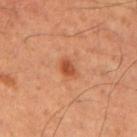Findings:
– follow-up — catalogued during a skin exam; not biopsied
– acquisition — total-body-photography crop, ~15 mm field of view
– body site — the arm
– lesion diameter — ≈2.5 mm
– subject — male, aged 53 to 57
– TBP lesion metrics — an average lesion color of about L≈50 a*≈29 b*≈38 (CIELAB), a lesion–skin lightness drop of about 10, and a normalized lesion–skin contrast near 7.5; a border-irregularity index near 2/10, internal color variation of about 2 on a 0–10 scale, and peripheral color asymmetry of about 0.5
– illumination — cross-polarized